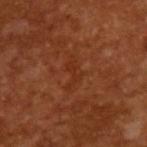A roughly 15 mm field-of-view crop from a total-body skin photograph. A male patient, aged approximately 65. Captured under cross-polarized illumination. Longest diameter approximately 4 mm. An algorithmic analysis of the crop reported a lesion color around L≈31 a*≈26 b*≈32 in CIELAB, a lesion–skin lightness drop of about 5, and a normalized border contrast of about 4.5. The analysis additionally found a classifier nevus-likeness of about 0/100.